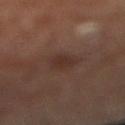Captured during whole-body skin photography for melanoma surveillance; the lesion was not biopsied.
Automated image analysis of the tile measured a footprint of about 5.5 mm² and two-axis asymmetry of about 0.25. The analysis additionally found border irregularity of about 2.5 on a 0–10 scale, internal color variation of about 2 on a 0–10 scale, and a peripheral color-asymmetry measure near 0.5.
The lesion is on the right lower leg.
A patient in their mid- to late 60s.
Captured under cross-polarized illumination.
Measured at roughly 3.5 mm in maximum diameter.
A 15 mm close-up extracted from a 3D total-body photography capture.